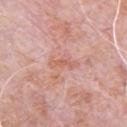Clinical impression:
Imaged during a routine full-body skin examination; the lesion was not biopsied and no histopathology is available.
Background:
A male subject aged approximately 80. Longest diameter approximately 3 mm. This is a white-light tile. A region of skin cropped from a whole-body photographic capture, roughly 15 mm wide. On the chest.